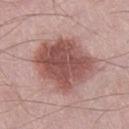Part of a total-body skin-imaging series; this lesion was reviewed on a skin check and was not flagged for biopsy. A male patient aged 53–57. This is a white-light tile. From the right thigh. The recorded lesion diameter is about 7.5 mm. A 15 mm crop from a total-body photograph taken for skin-cancer surveillance.